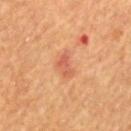notes = catalogued during a skin exam; not biopsied | patient = male, in their mid-50s | image = 15 mm crop, total-body photography | lighting = cross-polarized illumination | TBP lesion metrics = an area of roughly 4.5 mm², an eccentricity of roughly 0.8, and two-axis asymmetry of about 0.4; an automated nevus-likeness rating near 0 out of 100 and a detector confidence of about 100 out of 100 that the crop contains a lesion | location = the upper back | lesion diameter = about 3 mm.